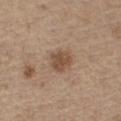Recorded during total-body skin imaging; not selected for excision or biopsy.
On the left thigh.
The subject is a male aged around 70.
A 15 mm close-up extracted from a 3D total-body photography capture.